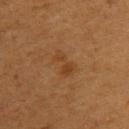biopsy status=imaged on a skin check; not biopsied | patient=male, aged around 55 | image source=~15 mm tile from a whole-body skin photo | site=the upper back | lesion diameter=about 3 mm | illumination=cross-polarized illumination.